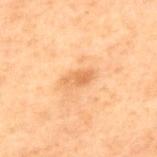Part of a total-body skin-imaging series; this lesion was reviewed on a skin check and was not flagged for biopsy. The lesion-visualizer software estimated a lesion area of about 5 mm², a shape eccentricity near 0.85, and two-axis asymmetry of about 0.25. And it measured a lesion color around L≈52 a*≈19 b*≈35 in CIELAB, a lesion–skin lightness drop of about 7, and a lesion-to-skin contrast of about 5.5 (normalized; higher = more distinct). The subject is a male about 70 years old. Cropped from a whole-body photographic skin survey; the tile spans about 15 mm. From the upper back.